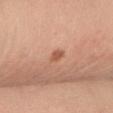Part of a total-body skin-imaging series; this lesion was reviewed on a skin check and was not flagged for biopsy. The tile uses cross-polarized illumination. Cropped from a whole-body photographic skin survey; the tile spans about 15 mm. From the right forearm. A female patient, aged around 35.